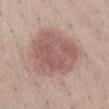Imaged during a routine full-body skin examination; the lesion was not biopsied and no histopathology is available.
Approximately 6.5 mm at its widest.
This is a white-light tile.
The lesion-visualizer software estimated an area of roughly 31 mm² and two-axis asymmetry of about 0.2. The analysis additionally found a lesion color around L≈56 a*≈20 b*≈22 in CIELAB and about 10 CIELAB-L* units darker than the surrounding skin. The analysis additionally found a within-lesion color-variation index near 3.5/10 and peripheral color asymmetry of about 1.
A female subject, in their mid- to late 60s.
Located on the front of the torso.
A 15 mm crop from a total-body photograph taken for skin-cancer surveillance.